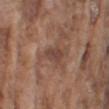A male subject, aged 73 to 77. The lesion's longest dimension is about 3.5 mm. Cropped from a total-body skin-imaging series; the visible field is about 15 mm. From the right upper arm.